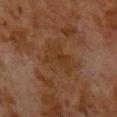Assessment: Recorded during total-body skin imaging; not selected for excision or biopsy. Image and clinical context: The subject is a male aged 78–82. A lesion tile, about 15 mm wide, cut from a 3D total-body photograph. The lesion-visualizer software estimated internal color variation of about 2 on a 0–10 scale and peripheral color asymmetry of about 0.5. Longest diameter approximately 3.5 mm. From the back. The tile uses cross-polarized illumination.